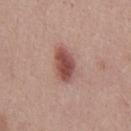Clinical impression:
The lesion was tiled from a total-body skin photograph and was not biopsied.
Context:
The lesion is on the chest. A male patient aged approximately 25. A roughly 15 mm field-of-view crop from a total-body skin photograph.